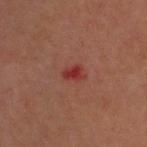– biopsy status · imaged on a skin check; not biopsied
– diameter · ≈2.5 mm
– lighting · cross-polarized illumination
– image-analysis metrics · a footprint of about 3 mm² and two-axis asymmetry of about 0.4; a border-irregularity index near 3.5/10; a classifier nevus-likeness of about 0/100
– patient · male, aged approximately 70
– anatomic site · the head or neck
– image · ~15 mm crop, total-body skin-cancer survey